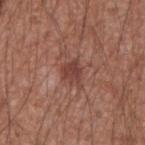notes = no biopsy performed (imaged during a skin exam)
patient = male, aged 58–62
lesion diameter = ~2.5 mm (longest diameter)
anatomic site = the right forearm
acquisition = 15 mm crop, total-body photography
illumination = white-light illumination